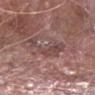follow-up=total-body-photography surveillance lesion; no biopsy | lighting=white-light illumination | location=the left lower leg | patient=male, aged approximately 65 | diameter=~5 mm (longest diameter) | imaging modality=total-body-photography crop, ~15 mm field of view.